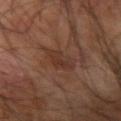Imaged during a routine full-body skin examination; the lesion was not biopsied and no histopathology is available. The subject is a male aged 58 to 62. Cropped from a total-body skin-imaging series; the visible field is about 15 mm. Located on the right arm. The total-body-photography lesion software estimated a lesion area of about 5.5 mm², an outline eccentricity of about 0.6 (0 = round, 1 = elongated), and two-axis asymmetry of about 0.3. The analysis additionally found a mean CIELAB color near L≈32 a*≈19 b*≈25, a lesion–skin lightness drop of about 6, and a normalized lesion–skin contrast near 5.5. And it measured a border-irregularity index near 3/10. The analysis additionally found a classifier nevus-likeness of about 0/100 and a detector confidence of about 100 out of 100 that the crop contains a lesion. Longest diameter approximately 3 mm. Captured under cross-polarized illumination.